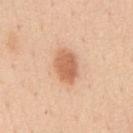Q: Is there a histopathology result?
A: no biopsy performed (imaged during a skin exam)
Q: How was the tile lit?
A: white-light
Q: Where on the body is the lesion?
A: the chest
Q: Patient demographics?
A: male, roughly 50 years of age
Q: How was this image acquired?
A: 15 mm crop, total-body photography
Q: What is the lesion's diameter?
A: ~4 mm (longest diameter)